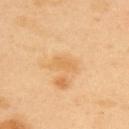Assessment:
Recorded during total-body skin imaging; not selected for excision or biopsy.
Context:
Imaged with cross-polarized lighting. About 3 mm across. On the upper back. A 15 mm crop from a total-body photograph taken for skin-cancer surveillance. A male patient, aged around 40.